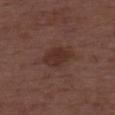The patient is a male aged around 50.
Imaged with white-light lighting.
A lesion tile, about 15 mm wide, cut from a 3D total-body photograph.